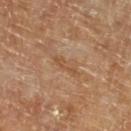Recorded during total-body skin imaging; not selected for excision or biopsy.
A male patient, in their mid-80s or older.
Cropped from a total-body skin-imaging series; the visible field is about 15 mm.
The tile uses cross-polarized illumination.
Located on the leg.
The total-body-photography lesion software estimated an outline eccentricity of about 0.95 (0 = round, 1 = elongated). The analysis additionally found an average lesion color of about L≈49 a*≈20 b*≈33 (CIELAB) and a lesion–skin lightness drop of about 6.